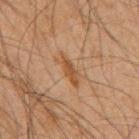notes: total-body-photography surveillance lesion; no biopsy | subject: male, in their mid- to late 60s | anatomic site: the mid back | illumination: cross-polarized illumination | imaging modality: 15 mm crop, total-body photography | automated metrics: a border-irregularity index near 4.5/10 and a peripheral color-asymmetry measure near 0.5.